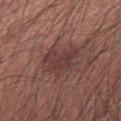Clinical impression:
No biopsy was performed on this lesion — it was imaged during a full skin examination and was not determined to be concerning.
Context:
A close-up tile cropped from a whole-body skin photograph, about 15 mm across. A male patient aged 28 to 32. About 5 mm across. From the arm. This is a white-light tile.